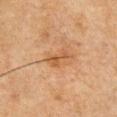Q: Who is the patient?
A: male, aged 48–52
Q: What is the anatomic site?
A: the front of the torso
Q: What kind of image is this?
A: total-body-photography crop, ~15 mm field of view
Q: Automated lesion metrics?
A: an area of roughly 3.5 mm²; border irregularity of about 5 on a 0–10 scale, a within-lesion color-variation index near 1.5/10, and radial color variation of about 0.5; an automated nevus-likeness rating near 0 out of 100
Q: What lighting was used for the tile?
A: cross-polarized